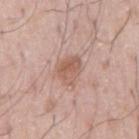Clinical impression:
This lesion was catalogued during total-body skin photography and was not selected for biopsy.
Clinical summary:
This image is a 15 mm lesion crop taken from a total-body photograph. The lesion's longest dimension is about 3 mm. Imaged with white-light lighting. A male subject, aged approximately 55.